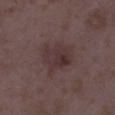Context:
A 15 mm crop from a total-body photograph taken for skin-cancer surveillance. An algorithmic analysis of the crop reported an area of roughly 11 mm², an outline eccentricity of about 0.65 (0 = round, 1 = elongated), and a shape-asymmetry score of about 0.25 (0 = symmetric). It also reported a border-irregularity index near 3/10 and a color-variation rating of about 4/10. About 4.5 mm across. A female subject, aged 33–37. The lesion is on the right lower leg. Imaged with white-light lighting.